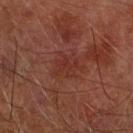Findings:
– workup: imaged on a skin check; not biopsied
– subject: male, aged 73–77
– size: ≈3 mm
– tile lighting: cross-polarized illumination
– image source: ~15 mm tile from a whole-body skin photo
– automated lesion analysis: border irregularity of about 8 on a 0–10 scale, internal color variation of about 1 on a 0–10 scale, and peripheral color asymmetry of about 0.5
– body site: the arm